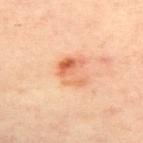{"biopsy_status": "not biopsied; imaged during a skin examination", "automated_metrics": {"area_mm2_approx": 7.5, "shape_asymmetry": 0.45, "vs_skin_darker_L": 11.0, "nevus_likeness_0_100": 65, "lesion_detection_confidence_0_100": 100}, "lighting": "cross-polarized", "image": {"source": "total-body photography crop", "field_of_view_mm": 15}, "patient": {"sex": "female", "age_approx": 40}, "site": "back"}